About 3 mm across; this image is a 15 mm lesion crop taken from a total-body photograph; from the right thigh; the subject is a male in their mid- to late 30s; imaged with cross-polarized lighting.
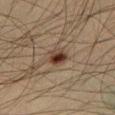The lesion was biopsied, and histopathology showed a dysplastic (Clark) nevus.